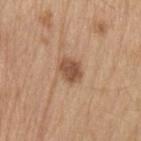biopsy status — imaged on a skin check; not biopsied
location — the left upper arm
acquisition — ~15 mm crop, total-body skin-cancer survey
subject — male, aged 68 to 72